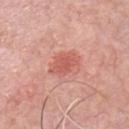* workup: catalogued during a skin exam; not biopsied
* image: ~15 mm tile from a whole-body skin photo
* lighting: white-light illumination
* automated lesion analysis: a mean CIELAB color near L≈59 a*≈30 b*≈30, about 9 CIELAB-L* units darker than the surrounding skin, and a normalized lesion–skin contrast near 6.5
* location: the chest
* diameter: ≈4.5 mm
* patient: male, in their mid- to late 40s